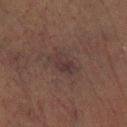Acquisition and patient details:
The lesion-visualizer software estimated a footprint of about 6 mm², an eccentricity of roughly 0.75, and a symmetry-axis asymmetry near 0.25. And it measured a mean CIELAB color near L≈26 a*≈13 b*≈15 and a lesion–skin lightness drop of about 4. It also reported a border-irregularity index near 3/10, a within-lesion color-variation index near 3.5/10, and peripheral color asymmetry of about 1. A roughly 15 mm field-of-view crop from a total-body skin photograph. Located on the leg. Longest diameter approximately 3.5 mm. The subject is a male approximately 75 years of age. This is a cross-polarized tile.